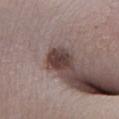This lesion was catalogued during total-body skin photography and was not selected for biopsy.
The lesion is located on the leg.
The patient is a female about 20 years old.
A region of skin cropped from a whole-body photographic capture, roughly 15 mm wide.